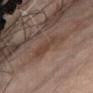Assessment:
Captured during whole-body skin photography for melanoma surveillance; the lesion was not biopsied.
Image and clinical context:
About 4 mm across. Captured under cross-polarized illumination. Located on the abdomen. An algorithmic analysis of the crop reported a lesion area of about 7 mm², a shape eccentricity near 0.85, and a symmetry-axis asymmetry near 0.25. The software also gave border irregularity of about 4 on a 0–10 scale and a peripheral color-asymmetry measure near 0.5. The analysis additionally found a classifier nevus-likeness of about 0/100 and a lesion-detection confidence of about 100/100. A female subject about 80 years old. A 15 mm close-up tile from a total-body photography series done for melanoma screening.